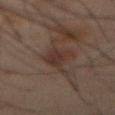Assessment: No biopsy was performed on this lesion — it was imaged during a full skin examination and was not determined to be concerning. Context: The subject is a male roughly 70 years of age. A roughly 15 mm field-of-view crop from a total-body skin photograph. The lesion is located on the mid back. Captured under cross-polarized illumination. Approximately 4.5 mm at its widest. The total-body-photography lesion software estimated a footprint of about 7 mm² and a symmetry-axis asymmetry near 0.35. The software also gave border irregularity of about 4.5 on a 0–10 scale, a color-variation rating of about 1.5/10, and radial color variation of about 0.5.